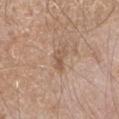  biopsy_status: not biopsied; imaged during a skin examination
  patient:
    sex: male
    age_approx: 65
  image:
    source: total-body photography crop
    field_of_view_mm: 15
  site: right forearm